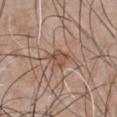Q: Lesion location?
A: the chest
Q: What lighting was used for the tile?
A: white-light
Q: What is the lesion's diameter?
A: about 3 mm
Q: What is the imaging modality?
A: ~15 mm tile from a whole-body skin photo
Q: What are the patient's age and sex?
A: male, aged 48–52